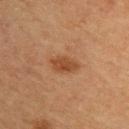This lesion was catalogued during total-body skin photography and was not selected for biopsy. This is a cross-polarized tile. A 15 mm close-up extracted from a 3D total-body photography capture. A male patient, aged around 60. From the upper back. The lesion's longest dimension is about 3.5 mm.